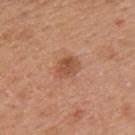Impression:
No biopsy was performed on this lesion — it was imaged during a full skin examination and was not determined to be concerning.
Background:
The lesion is on the left upper arm. Approximately 2.5 mm at its widest. The subject is a female aged 38–42. A region of skin cropped from a whole-body photographic capture, roughly 15 mm wide. Imaged with white-light lighting.